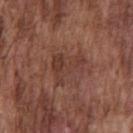Assessment: No biopsy was performed on this lesion — it was imaged during a full skin examination and was not determined to be concerning. Clinical summary: The subject is a male approximately 75 years of age. The total-body-photography lesion software estimated about 7 CIELAB-L* units darker than the surrounding skin. On the chest. A close-up tile cropped from a whole-body skin photograph, about 15 mm across. The lesion's longest dimension is about 4.5 mm.